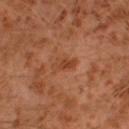No biopsy was performed on this lesion — it was imaged during a full skin examination and was not determined to be concerning. On the left leg. A male patient aged approximately 30. A close-up tile cropped from a whole-body skin photograph, about 15 mm across.